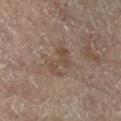Assessment:
Captured during whole-body skin photography for melanoma surveillance; the lesion was not biopsied.
Clinical summary:
A close-up tile cropped from a whole-body skin photograph, about 15 mm across. Approximately 3.5 mm at its widest. From the left leg. A female subject, about 80 years old. The total-body-photography lesion software estimated a lesion color around L≈41 a*≈13 b*≈23 in CIELAB, roughly 6 lightness units darker than nearby skin, and a normalized border contrast of about 6. The software also gave border irregularity of about 7.5 on a 0–10 scale, a color-variation rating of about 1.5/10, and peripheral color asymmetry of about 0.5. The software also gave a nevus-likeness score of about 0/100.